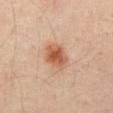<record>
<biopsy_status>not biopsied; imaged during a skin examination</biopsy_status>
<lighting>cross-polarized</lighting>
<patient>
  <sex>male</sex>
  <age_approx>65</age_approx>
</patient>
<site>abdomen</site>
<lesion_size>
  <long_diameter_mm_approx>4.0</long_diameter_mm_approx>
</lesion_size>
<automated_metrics>
  <cielab_L>53</cielab_L>
  <cielab_a>22</cielab_a>
  <cielab_b>32</cielab_b>
  <vs_skin_darker_L>12.0</vs_skin_darker_L>
  <border_irregularity_0_10>2.0</border_irregularity_0_10>
  <color_variation_0_10>4.5</color_variation_0_10>
  <peripheral_color_asymmetry>1.5</peripheral_color_asymmetry>
  <nevus_likeness_0_100>100</nevus_likeness_0_100>
</automated_metrics>
<image>
  <source>total-body photography crop</source>
  <field_of_view_mm>15</field_of_view_mm>
</image>
</record>